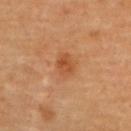Findings:
* subject · female, in their 60s
* anatomic site · the back
* image · 15 mm crop, total-body photography